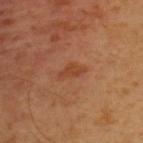No biopsy was performed on this lesion — it was imaged during a full skin examination and was not determined to be concerning. Imaged with cross-polarized lighting. A region of skin cropped from a whole-body photographic capture, roughly 15 mm wide. The lesion is located on the upper back. About 3 mm across. The subject is a male approximately 30 years of age.